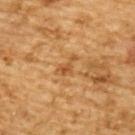Notes:
- location: the upper back
- patient: male, aged around 85 or older
- image: ~15 mm tile from a whole-body skin photo